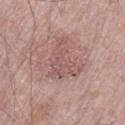Imaged during a routine full-body skin examination; the lesion was not biopsied and no histopathology is available. The lesion is on the left thigh. A close-up tile cropped from a whole-body skin photograph, about 15 mm across. Longest diameter approximately 5 mm. A male subject, roughly 70 years of age. Captured under white-light illumination.